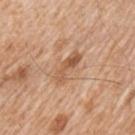This lesion was catalogued during total-body skin photography and was not selected for biopsy.
A 15 mm close-up tile from a total-body photography series done for melanoma screening.
A male subject in their mid- to late 60s.
From the left upper arm.